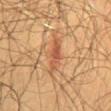Q: Is there a histopathology result?
A: no biopsy performed (imaged during a skin exam)
Q: What are the patient's age and sex?
A: male, aged around 60
Q: How was this image acquired?
A: 15 mm crop, total-body photography
Q: What is the lesion's diameter?
A: ≈4.5 mm
Q: How was the tile lit?
A: cross-polarized illumination
Q: What did automated image analysis measure?
A: a border-irregularity rating of about 4/10, a color-variation rating of about 3.5/10, and a peripheral color-asymmetry measure near 1; lesion-presence confidence of about 100/100
Q: What is the anatomic site?
A: the abdomen